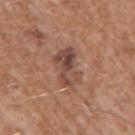The lesion's longest dimension is about 5 mm.
A roughly 15 mm field-of-view crop from a total-body skin photograph.
The tile uses white-light illumination.
The patient is a male aged 58–62.
The lesion is on the left upper arm.